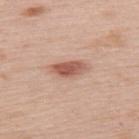Part of a total-body skin-imaging series; this lesion was reviewed on a skin check and was not flagged for biopsy. Longest diameter approximately 4.5 mm. Located on the upper back. The subject is a female in their mid- to late 60s. Captured under white-light illumination. Cropped from a whole-body photographic skin survey; the tile spans about 15 mm.